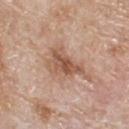Clinical impression: No biopsy was performed on this lesion — it was imaged during a full skin examination and was not determined to be concerning. Image and clinical context: The recorded lesion diameter is about 6 mm. An algorithmic analysis of the crop reported an area of roughly 13 mm², an outline eccentricity of about 0.8 (0 = round, 1 = elongated), and a shape-asymmetry score of about 0.45 (0 = symmetric). The software also gave a mean CIELAB color near L≈56 a*≈20 b*≈29 and a lesion-to-skin contrast of about 7 (normalized; higher = more distinct). And it measured lesion-presence confidence of about 100/100. The lesion is on the back. A male subject approximately 80 years of age. A lesion tile, about 15 mm wide, cut from a 3D total-body photograph. The tile uses white-light illumination.